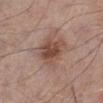biopsy_status: not biopsied; imaged during a skin examination
lesion_size:
  long_diameter_mm_approx: 4.0
site: left lower leg
patient:
  sex: male
  age_approx: 55
image:
  source: total-body photography crop
  field_of_view_mm: 15
lighting: white-light